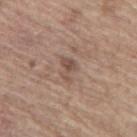Q: Is there a histopathology result?
A: catalogued during a skin exam; not biopsied
Q: How was the tile lit?
A: white-light illumination
Q: Lesion location?
A: the left thigh
Q: Automated lesion metrics?
A: a nevus-likeness score of about 0/100 and a lesion-detection confidence of about 100/100
Q: Who is the patient?
A: male, aged around 70
Q: What kind of image is this?
A: ~15 mm crop, total-body skin-cancer survey